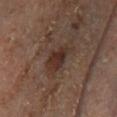site: right lower leg
lesion_size:
  long_diameter_mm_approx: 5.0
image:
  source: total-body photography crop
  field_of_view_mm: 15
patient:
  sex: female
  age_approx: 50
lighting: cross-polarized